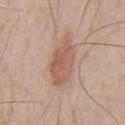Part of a total-body skin-imaging series; this lesion was reviewed on a skin check and was not flagged for biopsy. On the chest. The subject is a male aged approximately 50. A close-up tile cropped from a whole-body skin photograph, about 15 mm across.